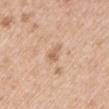Case summary:
• biopsy status — catalogued during a skin exam; not biopsied
• patient — female, roughly 40 years of age
• anatomic site — the right upper arm
• image source — total-body-photography crop, ~15 mm field of view
• tile lighting — white-light illumination